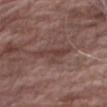Imaged during a routine full-body skin examination; the lesion was not biopsied and no histopathology is available.
Located on the right thigh.
Longest diameter approximately 4.5 mm.
Automated tile analysis of the lesion measured a mean CIELAB color near L≈40 a*≈19 b*≈21 and roughly 8 lightness units darker than nearby skin. And it measured border irregularity of about 8 on a 0–10 scale and radial color variation of about 0.
A male patient, approximately 80 years of age.
A close-up tile cropped from a whole-body skin photograph, about 15 mm across.